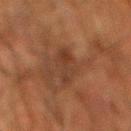The lesion was tiled from a total-body skin photograph and was not biopsied. Captured under cross-polarized illumination. The total-body-photography lesion software estimated an area of roughly 7 mm², an outline eccentricity of about 0.95 (0 = round, 1 = elongated), and two-axis asymmetry of about 0.25. The analysis additionally found a border-irregularity index near 4.5/10 and a color-variation rating of about 3.5/10. Located on the mid back. Measured at roughly 5.5 mm in maximum diameter. A 15 mm close-up tile from a total-body photography series done for melanoma screening. A male patient aged approximately 80.